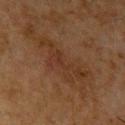Assessment:
Captured during whole-body skin photography for melanoma surveillance; the lesion was not biopsied.
Image and clinical context:
The lesion is located on the left upper arm. A 15 mm close-up tile from a total-body photography series done for melanoma screening. A male patient about 65 years old.